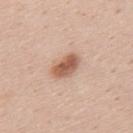Assessment:
Captured during whole-body skin photography for melanoma surveillance; the lesion was not biopsied.
Image and clinical context:
On the back. A male patient, roughly 45 years of age. A 15 mm close-up extracted from a 3D total-body photography capture.